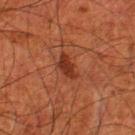workup=total-body-photography surveillance lesion; no biopsy | illumination=cross-polarized illumination | image-analysis metrics=a nevus-likeness score of about 55/100 and lesion-presence confidence of about 100/100 | anatomic site=the left lower leg | subject=male, approximately 80 years of age | imaging modality=~15 mm crop, total-body skin-cancer survey.